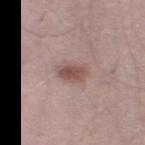Findings:
• follow-up: imaged on a skin check; not biopsied
• tile lighting: white-light illumination
• lesion size: ≈3 mm
• subject: male, aged around 30
• image-analysis metrics: an eccentricity of roughly 0.7 and a shape-asymmetry score of about 0.25 (0 = symmetric); a mean CIELAB color near L≈51 a*≈19 b*≈21, roughly 11 lightness units darker than nearby skin, and a lesion-to-skin contrast of about 8 (normalized; higher = more distinct); peripheral color asymmetry of about 1; a nevus-likeness score of about 85/100 and a detector confidence of about 100 out of 100 that the crop contains a lesion
• imaging modality: total-body-photography crop, ~15 mm field of view